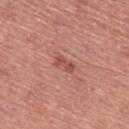{
  "biopsy_status": "not biopsied; imaged during a skin examination",
  "patient": {
    "sex": "male",
    "age_approx": 50
  },
  "image": {
    "source": "total-body photography crop",
    "field_of_view_mm": 15
  },
  "site": "left thigh"
}